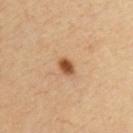Imaged during a routine full-body skin examination; the lesion was not biopsied and no histopathology is available.
A 15 mm crop from a total-body photograph taken for skin-cancer surveillance.
The subject is a male roughly 65 years of age.
The lesion is on the front of the torso.